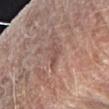workup=total-body-photography surveillance lesion; no biopsy
anatomic site=the right forearm
lighting=white-light illumination
patient=male, aged 78 to 82
imaging modality=15 mm crop, total-body photography
lesion size=~3.5 mm (longest diameter)